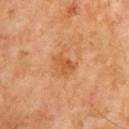Findings:
- biopsy status — total-body-photography surveillance lesion; no biopsy
- subject — male, aged 63 to 67
- acquisition — 15 mm crop, total-body photography
- size — about 3 mm
- lighting — cross-polarized
- image-analysis metrics — a footprint of about 4.5 mm², an eccentricity of roughly 0.8, and a shape-asymmetry score of about 0.25 (0 = symmetric); a lesion–skin lightness drop of about 8 and a lesion-to-skin contrast of about 6 (normalized; higher = more distinct)